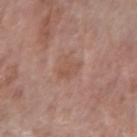Q: Was this lesion biopsied?
A: catalogued during a skin exam; not biopsied
Q: How was this image acquired?
A: ~15 mm crop, total-body skin-cancer survey
Q: Who is the patient?
A: female, aged 58 to 62
Q: What did automated image analysis measure?
A: about 6 CIELAB-L* units darker than the surrounding skin and a lesion-to-skin contrast of about 5 (normalized; higher = more distinct); a border-irregularity index near 3/10 and a within-lesion color-variation index near 1.5/10
Q: What lighting was used for the tile?
A: white-light illumination
Q: What is the anatomic site?
A: the right forearm